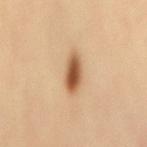• workup — imaged on a skin check; not biopsied
• site — the mid back
• imaging modality — ~15 mm tile from a whole-body skin photo
• patient — male, approximately 55 years of age